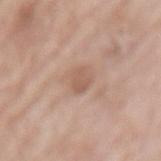Assessment: No biopsy was performed on this lesion — it was imaged during a full skin examination and was not determined to be concerning. Acquisition and patient details: The lesion is on the mid back. A 15 mm crop from a total-body photograph taken for skin-cancer surveillance. Imaged with white-light lighting. Approximately 2.5 mm at its widest. A female subject about 75 years old.